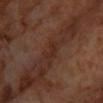Acquisition and patient details:
A male subject aged approximately 60. A roughly 15 mm field-of-view crop from a total-body skin photograph. The recorded lesion diameter is about 2.5 mm. On the front of the torso.